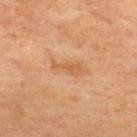Impression: No biopsy was performed on this lesion — it was imaged during a full skin examination and was not determined to be concerning.